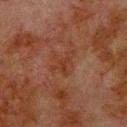{"biopsy_status": "not biopsied; imaged during a skin examination", "image": {"source": "total-body photography crop", "field_of_view_mm": 15}, "site": "upper back", "patient": {"sex": "male", "age_approx": 80}, "lesion_size": {"long_diameter_mm_approx": 3.5}, "lighting": "cross-polarized"}